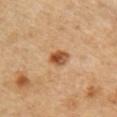Case summary:
- subject · male, in their mid- to late 50s
- location · the right upper arm
- lesion diameter · about 3.5 mm
- image · 15 mm crop, total-body photography
- automated lesion analysis · a nevus-likeness score of about 95/100 and a detector confidence of about 100 out of 100 that the crop contains a lesion